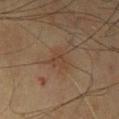<record>
<biopsy_status>not biopsied; imaged during a skin examination</biopsy_status>
<lesion_size>
  <long_diameter_mm_approx>2.5</long_diameter_mm_approx>
</lesion_size>
<image>
  <source>total-body photography crop</source>
  <field_of_view_mm>15</field_of_view_mm>
</image>
<patient>
  <sex>male</sex>
  <age_approx>75</age_approx>
</patient>
<lighting>cross-polarized</lighting>
<automated_metrics>
  <area_mm2_approx>4.5</area_mm2_approx>
  <shape_asymmetry>0.35</shape_asymmetry>
  <border_irregularity_0_10>4.0</border_irregularity_0_10>
  <color_variation_0_10>1.5</color_variation_0_10>
  <peripheral_color_asymmetry>0.5</peripheral_color_asymmetry>
</automated_metrics>
<site>right upper arm</site>
</record>